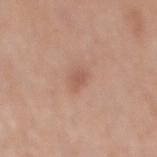Impression:
Captured during whole-body skin photography for melanoma surveillance; the lesion was not biopsied.
Clinical summary:
The patient is a male aged 68 to 72. Located on the mid back. Cropped from a total-body skin-imaging series; the visible field is about 15 mm.